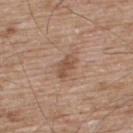Notes:
* workup — imaged on a skin check; not biopsied
* diameter — ~3 mm (longest diameter)
* automated metrics — a footprint of about 4 mm², a shape eccentricity near 0.85, and a symmetry-axis asymmetry near 0.4; border irregularity of about 4 on a 0–10 scale and a peripheral color-asymmetry measure near 0.5; a nevus-likeness score of about 15/100 and a detector confidence of about 100 out of 100 that the crop contains a lesion
* body site — the upper back
* subject — male, in their mid-60s
* image source — total-body-photography crop, ~15 mm field of view